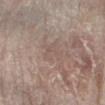Impression:
The lesion was tiled from a total-body skin photograph and was not biopsied.
Background:
A roughly 15 mm field-of-view crop from a total-body skin photograph. The patient is a male approximately 80 years of age. About 3 mm across. The lesion-visualizer software estimated a lesion color around L≈54 a*≈15 b*≈22 in CIELAB, about 4 CIELAB-L* units darker than the surrounding skin, and a lesion-to-skin contrast of about 3 (normalized; higher = more distinct). It also reported a nevus-likeness score of about 0/100 and a detector confidence of about 90 out of 100 that the crop contains a lesion. The lesion is on the right forearm. This is a white-light tile.